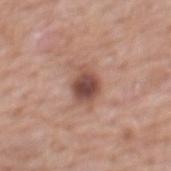On the mid back. Cropped from a whole-body photographic skin survey; the tile spans about 15 mm. The subject is a female aged around 60. Longest diameter approximately 5 mm.